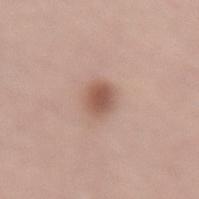notes: catalogued during a skin exam; not biopsied
lesion diameter: ≈2.5 mm
patient: female, in their mid- to late 60s
tile lighting: white-light illumination
acquisition: total-body-photography crop, ~15 mm field of view
site: the lower back